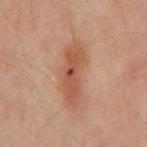follow-up — catalogued during a skin exam; not biopsied
image-analysis metrics — a footprint of about 13 mm² and an outline eccentricity of about 0.95 (0 = round, 1 = elongated); a lesion–skin lightness drop of about 8
location — the mid back
lighting — cross-polarized
patient — male, about 45 years old
imaging modality — ~15 mm tile from a whole-body skin photo